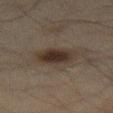  biopsy_status: not biopsied; imaged during a skin examination
  patient:
    sex: male
    age_approx: 60
  image:
    source: total-body photography crop
    field_of_view_mm: 15
  site: abdomen
  lesion_size:
    long_diameter_mm_approx: 4.0